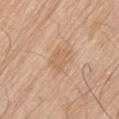Imaged during a routine full-body skin examination; the lesion was not biopsied and no histopathology is available. A lesion tile, about 15 mm wide, cut from a 3D total-body photograph. The patient is a male roughly 80 years of age. Imaged with white-light lighting. On the left thigh.